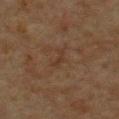Assessment: Part of a total-body skin-imaging series; this lesion was reviewed on a skin check and was not flagged for biopsy. Context: This is a cross-polarized tile. A male subject roughly 60 years of age. A region of skin cropped from a whole-body photographic capture, roughly 15 mm wide. Located on the chest. An algorithmic analysis of the crop reported a lesion color around L≈29 a*≈14 b*≈24 in CIELAB and a normalized lesion–skin contrast near 4.5. About 2.5 mm across.